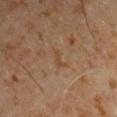biopsy status — no biopsy performed (imaged during a skin exam)
image — ~15 mm crop, total-body skin-cancer survey
automated lesion analysis — a lesion area of about 2 mm², a shape eccentricity near 0.95, and two-axis asymmetry of about 0.35; a lesion color around L≈42 a*≈17 b*≈31 in CIELAB, about 5 CIELAB-L* units darker than the surrounding skin, and a normalized lesion–skin contrast near 5.5
lesion diameter — about 2.5 mm
illumination — cross-polarized illumination
patient — male, aged around 60
body site — the arm